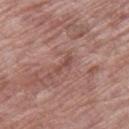Recorded during total-body skin imaging; not selected for excision or biopsy. Automated tile analysis of the lesion measured an eccentricity of roughly 0.95 and two-axis asymmetry of about 0.45. The software also gave roughly 8 lightness units darker than nearby skin and a normalized border contrast of about 6. And it measured a border-irregularity rating of about 5.5/10, a within-lesion color-variation index near 0/10, and a peripheral color-asymmetry measure near 0. And it measured an automated nevus-likeness rating near 0 out of 100 and lesion-presence confidence of about 95/100. About 2.5 mm across. The lesion is on the right thigh. The patient is a female about 70 years old. A region of skin cropped from a whole-body photographic capture, roughly 15 mm wide.